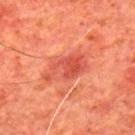• biopsy status · catalogued during a skin exam; not biopsied
• acquisition · ~15 mm crop, total-body skin-cancer survey
• lighting · cross-polarized
• patient · male, aged around 65
• size · ~5.5 mm (longest diameter)
• body site · the upper back